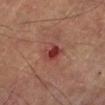<tbp_lesion>
  <patient>
    <sex>male</sex>
    <age_approx>55</age_approx>
  </patient>
  <lesion_size>
    <long_diameter_mm_approx>2.5</long_diameter_mm_approx>
  </lesion_size>
  <image>
    <source>total-body photography crop</source>
    <field_of_view_mm>15</field_of_view_mm>
  </image>
  <site>left lower leg</site>
  <automated_metrics>
    <area_mm2_approx>4.0</area_mm2_approx>
    <shape_asymmetry>0.3</shape_asymmetry>
    <cielab_L>33</cielab_L>
    <cielab_a>27</cielab_a>
    <cielab_b>23</cielab_b>
    <vs_skin_darker_L>10.0</vs_skin_darker_L>
    <vs_skin_contrast_norm>9.0</vs_skin_contrast_norm>
    <peripheral_color_asymmetry>0.5</peripheral_color_asymmetry>
  </automated_metrics>
</tbp_lesion>